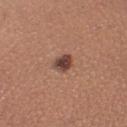Assessment:
No biopsy was performed on this lesion — it was imaged during a full skin examination and was not determined to be concerning.
Acquisition and patient details:
Automated image analysis of the tile measured a mean CIELAB color near L≈43 a*≈21 b*≈25, a lesion–skin lightness drop of about 14, and a normalized border contrast of about 11. It also reported a within-lesion color-variation index near 3/10 and a peripheral color-asymmetry measure near 0.5. And it measured a nevus-likeness score of about 85/100. The lesion is located on the left lower leg. A female patient roughly 30 years of age. Imaged with white-light lighting. Measured at roughly 2.5 mm in maximum diameter. A close-up tile cropped from a whole-body skin photograph, about 15 mm across.